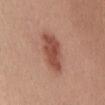Imaged during a routine full-body skin examination; the lesion was not biopsied and no histopathology is available. From the chest. A close-up tile cropped from a whole-body skin photograph, about 15 mm across. A female subject aged 43 to 47.